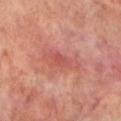biopsy status: no biopsy performed (imaged during a skin exam)
image source: total-body-photography crop, ~15 mm field of view
body site: the left lower leg
lighting: cross-polarized illumination
automated metrics: a lesion color around L≈52 a*≈31 b*≈27 in CIELAB, about 7 CIELAB-L* units darker than the surrounding skin, and a lesion-to-skin contrast of about 5 (normalized; higher = more distinct); a peripheral color-asymmetry measure near 0.5
subject: male, aged 68–72